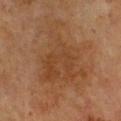Q: Was a biopsy performed?
A: catalogued during a skin exam; not biopsied
Q: Who is the patient?
A: female, aged around 60
Q: What is the anatomic site?
A: the chest
Q: How large is the lesion?
A: ≈12 mm
Q: Automated lesion metrics?
A: border irregularity of about 7.5 on a 0–10 scale, a within-lesion color-variation index near 4/10, and peripheral color asymmetry of about 1; a detector confidence of about 100 out of 100 that the crop contains a lesion
Q: How was the tile lit?
A: cross-polarized
Q: What is the imaging modality?
A: ~15 mm crop, total-body skin-cancer survey